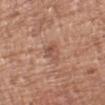Clinical impression: Imaged during a routine full-body skin examination; the lesion was not biopsied and no histopathology is available. Context: A 15 mm close-up extracted from a 3D total-body photography capture. A male subject, aged 63–67. Longest diameter approximately 3 mm. Located on the left upper arm.